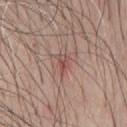follow-up: total-body-photography surveillance lesion; no biopsy
size: ~2.5 mm (longest diameter)
site: the chest
tile lighting: white-light
image source: ~15 mm crop, total-body skin-cancer survey
patient: male, approximately 65 years of age